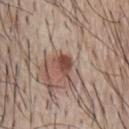Q: Was this lesion biopsied?
A: catalogued during a skin exam; not biopsied
Q: Patient demographics?
A: male, about 65 years old
Q: How was this image acquired?
A: total-body-photography crop, ~15 mm field of view
Q: Lesion location?
A: the chest